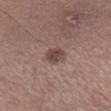– workup · imaged on a skin check; not biopsied
– patient · male, approximately 40 years of age
– site · the right lower leg
– image source · ~15 mm tile from a whole-body skin photo